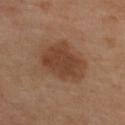Clinical impression:
Captured during whole-body skin photography for melanoma surveillance; the lesion was not biopsied.
Acquisition and patient details:
Cropped from a whole-body photographic skin survey; the tile spans about 15 mm. Approximately 5.5 mm at its widest. The subject is a female in their 40s. From the upper back.